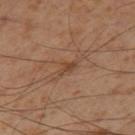No biopsy was performed on this lesion — it was imaged during a full skin examination and was not determined to be concerning. About 2.5 mm across. The lesion is on the leg. The tile uses cross-polarized illumination. A male patient, in their mid-50s. A roughly 15 mm field-of-view crop from a total-body skin photograph.